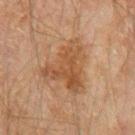Impression: Recorded during total-body skin imaging; not selected for excision or biopsy. Background: A male subject, roughly 45 years of age. From the right upper arm. A close-up tile cropped from a whole-body skin photograph, about 15 mm across. Captured under cross-polarized illumination. The lesion's longest dimension is about 6 mm. Automated tile analysis of the lesion measured an average lesion color of about L≈44 a*≈18 b*≈31 (CIELAB), roughly 8 lightness units darker than nearby skin, and a lesion-to-skin contrast of about 7 (normalized; higher = more distinct). The analysis additionally found a border-irregularity index near 6.5/10, internal color variation of about 4 on a 0–10 scale, and radial color variation of about 1.5.